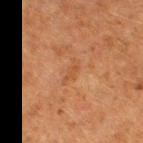Notes:
- biopsy status — imaged on a skin check; not biopsied
- illumination — cross-polarized
- subject — female, in their 50s
- site — the right thigh
- TBP lesion metrics — a lesion color around L≈42 a*≈21 b*≈33 in CIELAB and a lesion-to-skin contrast of about 4.5 (normalized; higher = more distinct); a border-irregularity rating of about 4.5/10, internal color variation of about 1 on a 0–10 scale, and peripheral color asymmetry of about 0
- imaging modality — 15 mm crop, total-body photography